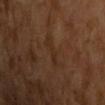Imaged during a routine full-body skin examination; the lesion was not biopsied and no histopathology is available.
The tile uses cross-polarized illumination.
Cropped from a whole-body photographic skin survey; the tile spans about 15 mm.
About 3.5 mm across.
A male patient in their mid-60s.